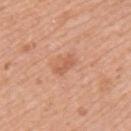biopsy status: imaged on a skin check; not biopsied
body site: the left upper arm
subject: female, aged approximately 40
automated metrics: border irregularity of about 4 on a 0–10 scale and radial color variation of about 0; a nevus-likeness score of about 5/100
image: total-body-photography crop, ~15 mm field of view
lesion diameter: ~2.5 mm (longest diameter)
tile lighting: white-light illumination